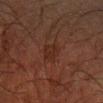<lesion>
  <biopsy_status>not biopsied; imaged during a skin examination</biopsy_status>
  <lesion_size>
    <long_diameter_mm_approx>3.0</long_diameter_mm_approx>
  </lesion_size>
  <site>right forearm</site>
  <automated_metrics>
    <area_mm2_approx>5.0</area_mm2_approx>
    <eccentricity>0.6</eccentricity>
    <cielab_L>22</cielab_L>
    <cielab_a>17</cielab_a>
    <cielab_b>22</cielab_b>
    <vs_skin_darker_L>4.0</vs_skin_darker_L>
    <vs_skin_contrast_norm>5.5</vs_skin_contrast_norm>
  </automated_metrics>
  <patient>
    <sex>male</sex>
    <age_approx>65</age_approx>
  </patient>
  <image>
    <source>total-body photography crop</source>
    <field_of_view_mm>15</field_of_view_mm>
  </image>
</lesion>